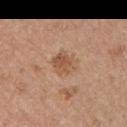notes = total-body-photography surveillance lesion; no biopsy | image = 15 mm crop, total-body photography | tile lighting = white-light illumination | location = the left upper arm | automated metrics = a lesion–skin lightness drop of about 9 and a normalized border contrast of about 6.5; an automated nevus-likeness rating near 35 out of 100 | size = ~3 mm (longest diameter) | patient = male, in their mid-50s.